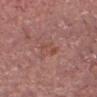Q: How was this image acquired?
A: ~15 mm tile from a whole-body skin photo
Q: Where on the body is the lesion?
A: the head or neck
Q: Who is the patient?
A: male, aged 63 to 67
Q: How large is the lesion?
A: ≈3 mm
Q: What did automated image analysis measure?
A: a mean CIELAB color near L≈48 a*≈24 b*≈26 and a normalized border contrast of about 5.5; a classifier nevus-likeness of about 0/100 and a detector confidence of about 100 out of 100 that the crop contains a lesion